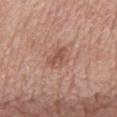Impression:
Part of a total-body skin-imaging series; this lesion was reviewed on a skin check and was not flagged for biopsy.
Clinical summary:
About 3 mm across. Automated image analysis of the tile measured border irregularity of about 2.5 on a 0–10 scale, a color-variation rating of about 3.5/10, and radial color variation of about 1.5. And it measured a classifier nevus-likeness of about 0/100. On the mid back. Cropped from a total-body skin-imaging series; the visible field is about 15 mm. The subject is a male in their mid-70s.